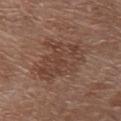Q: Was this lesion biopsied?
A: total-body-photography surveillance lesion; no biopsy
Q: Who is the patient?
A: female, in their 70s
Q: Illumination type?
A: white-light
Q: What is the anatomic site?
A: the upper back
Q: What is the lesion's diameter?
A: ~6.5 mm (longest diameter)
Q: What kind of image is this?
A: total-body-photography crop, ~15 mm field of view